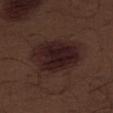<record>
  <biopsy_status>not biopsied; imaged during a skin examination</biopsy_status>
  <lesion_size>
    <long_diameter_mm_approx>7.5</long_diameter_mm_approx>
  </lesion_size>
  <lighting>white-light</lighting>
  <image>
    <source>total-body photography crop</source>
    <field_of_view_mm>15</field_of_view_mm>
  </image>
  <patient>
    <sex>male</sex>
    <age_approx>70</age_approx>
  </patient>
  <site>abdomen</site>
</record>